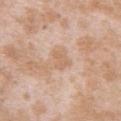notes — no biopsy performed (imaged during a skin exam) | site — the upper back | imaging modality — 15 mm crop, total-body photography | subject — female, roughly 25 years of age.